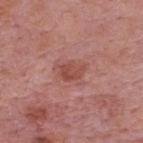This lesion was catalogued during total-body skin photography and was not selected for biopsy. About 3 mm across. A male patient, in their mid-70s. Located on the back. A close-up tile cropped from a whole-body skin photograph, about 15 mm across. Imaged with white-light lighting.